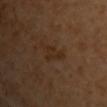Assessment:
Part of a total-body skin-imaging series; this lesion was reviewed on a skin check and was not flagged for biopsy.
Clinical summary:
A 15 mm close-up tile from a total-body photography series done for melanoma screening. A female subject. Imaged with cross-polarized lighting. Located on the upper back. About 3 mm across.